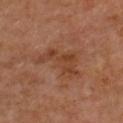Notes:
– follow-up: imaged on a skin check; not biopsied
– image source: 15 mm crop, total-body photography
– illumination: cross-polarized illumination
– body site: the chest
– lesion diameter: ≈6 mm
– subject: female, about 50 years old